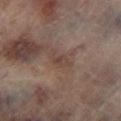Impression: Captured during whole-body skin photography for melanoma surveillance; the lesion was not biopsied. Context: On the left lower leg. The total-body-photography lesion software estimated a lesion area of about 3.5 mm², a shape eccentricity near 0.9, and a symmetry-axis asymmetry near 0.45. And it measured an average lesion color of about L≈39 a*≈16 b*≈22 (CIELAB), roughly 6 lightness units darker than nearby skin, and a normalized lesion–skin contrast near 5.5. This is a cross-polarized tile. About 3 mm across. Cropped from a whole-body photographic skin survey; the tile spans about 15 mm. A male patient roughly 65 years of age.